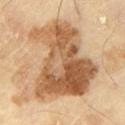follow-up=total-body-photography surveillance lesion; no biopsy | subject=male, roughly 65 years of age | imaging modality=~15 mm tile from a whole-body skin photo | anatomic site=the left thigh.